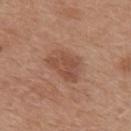Case summary:
• biopsy status: catalogued during a skin exam; not biopsied
• site: the back
• acquisition: ~15 mm crop, total-body skin-cancer survey
• illumination: white-light
• automated metrics: a lesion area of about 13 mm² and a shape eccentricity near 0.7; a lesion color around L≈50 a*≈21 b*≈29 in CIELAB and a lesion-to-skin contrast of about 6 (normalized; higher = more distinct); border irregularity of about 2.5 on a 0–10 scale, a within-lesion color-variation index near 3/10, and peripheral color asymmetry of about 1
• subject: male, about 30 years old
• lesion diameter: ~5 mm (longest diameter)